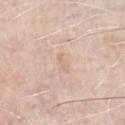The lesion was photographed on a routine skin check and not biopsied; there is no pathology result. Measured at roughly 2.5 mm in maximum diameter. A 15 mm crop from a total-body photograph taken for skin-cancer surveillance. From the chest. The tile uses white-light illumination. A male patient aged approximately 75.